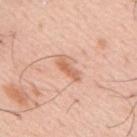Part of a total-body skin-imaging series; this lesion was reviewed on a skin check and was not flagged for biopsy. On the mid back. A male subject, roughly 55 years of age. A 15 mm close-up tile from a total-body photography series done for melanoma screening. Imaged with white-light lighting.